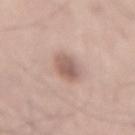Captured during whole-body skin photography for melanoma surveillance; the lesion was not biopsied. The patient is a male approximately 70 years of age. Captured under white-light illumination. On the mid back. A region of skin cropped from a whole-body photographic capture, roughly 15 mm wide. About 3.5 mm across.